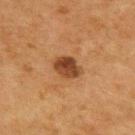No biopsy was performed on this lesion — it was imaged during a full skin examination and was not determined to be concerning. On the upper back. The lesion's longest dimension is about 3.5 mm. Captured under cross-polarized illumination. This image is a 15 mm lesion crop taken from a total-body photograph. Automated tile analysis of the lesion measured a lesion–skin lightness drop of about 12 and a lesion-to-skin contrast of about 10.5 (normalized; higher = more distinct). It also reported a border-irregularity index near 1.5/10 and peripheral color asymmetry of about 1.5. A male subject, in their mid- to late 70s.